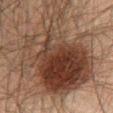Assessment: The lesion was tiled from a total-body skin photograph and was not biopsied. Context: The lesion is on the abdomen. This is a cross-polarized tile. The patient is a male aged 58–62. The recorded lesion diameter is about 12.5 mm. A 15 mm close-up tile from a total-body photography series done for melanoma screening.